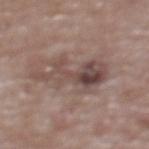follow-up = no biopsy performed (imaged during a skin exam)
subject = female, in their mid-60s
site = the upper back
illumination = white-light illumination
lesion diameter = ~8.5 mm (longest diameter)
imaging modality = ~15 mm crop, total-body skin-cancer survey